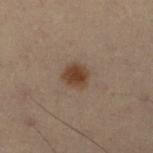The lesion was tiled from a total-body skin photograph and was not biopsied.
Approximately 3 mm at its widest.
The patient is a male aged 53–57.
The lesion is on the leg.
Cropped from a whole-body photographic skin survey; the tile spans about 15 mm.
This is a cross-polarized tile.
The total-body-photography lesion software estimated a lesion–skin lightness drop of about 9 and a lesion-to-skin contrast of about 9.5 (normalized; higher = more distinct). And it measured lesion-presence confidence of about 100/100.